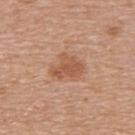Clinical impression:
No biopsy was performed on this lesion — it was imaged during a full skin examination and was not determined to be concerning.
Clinical summary:
Cropped from a total-body skin-imaging series; the visible field is about 15 mm. About 4 mm across. This is a white-light tile. Located on the upper back. The subject is a female aged 68–72.